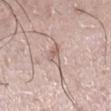No biopsy was performed on this lesion — it was imaged during a full skin examination and was not determined to be concerning.